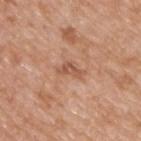Assessment: Captured during whole-body skin photography for melanoma surveillance; the lesion was not biopsied. Acquisition and patient details: A male subject, in their 50s. The tile uses white-light illumination. About 3 mm across. Automated image analysis of the tile measured a footprint of about 3.5 mm², a shape eccentricity near 0.85, and two-axis asymmetry of about 0.55. The software also gave border irregularity of about 5.5 on a 0–10 scale. The software also gave a nevus-likeness score of about 0/100 and a detector confidence of about 100 out of 100 that the crop contains a lesion. On the upper back. A lesion tile, about 15 mm wide, cut from a 3D total-body photograph.